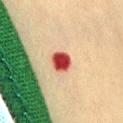The lesion was photographed on a routine skin check and not biopsied; there is no pathology result. A close-up tile cropped from a whole-body skin photograph, about 15 mm across. Measured at roughly 2.5 mm in maximum diameter. A female subject aged 38 to 42. Located on the abdomen.